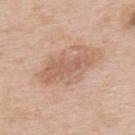Clinical impression: Recorded during total-body skin imaging; not selected for excision or biopsy. Clinical summary: The recorded lesion diameter is about 7 mm. The patient is a male aged approximately 65. The lesion is located on the upper back. Captured under white-light illumination. The total-body-photography lesion software estimated an area of roughly 17 mm², an outline eccentricity of about 0.85 (0 = round, 1 = elongated), and a symmetry-axis asymmetry near 0.3. The analysis additionally found a mean CIELAB color near L≈61 a*≈19 b*≈30, a lesion–skin lightness drop of about 9, and a normalized border contrast of about 6. And it measured border irregularity of about 5 on a 0–10 scale, a color-variation rating of about 3.5/10, and radial color variation of about 1. It also reported a classifier nevus-likeness of about 5/100 and a lesion-detection confidence of about 100/100. Cropped from a whole-body photographic skin survey; the tile spans about 15 mm.